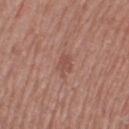{
  "biopsy_status": "not biopsied; imaged during a skin examination"
}